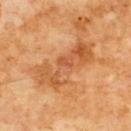Image and clinical context:
Imaged with cross-polarized lighting. Located on the chest. A lesion tile, about 15 mm wide, cut from a 3D total-body photograph. A male patient, aged 58 to 62. Automated image analysis of the tile measured a lesion area of about 19 mm², an outline eccentricity of about 0.9 (0 = round, 1 = elongated), and a symmetry-axis asymmetry near 0.35. It also reported a lesion color around L≈54 a*≈26 b*≈41 in CIELAB, about 9 CIELAB-L* units darker than the surrounding skin, and a lesion-to-skin contrast of about 6.5 (normalized; higher = more distinct). It also reported a border-irregularity index near 6/10, a within-lesion color-variation index near 7/10, and radial color variation of about 2. It also reported an automated nevus-likeness rating near 0 out of 100. The lesion's longest dimension is about 8 mm.